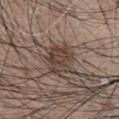This lesion was catalogued during total-body skin photography and was not selected for biopsy. An algorithmic analysis of the crop reported a lesion color around L≈41 a*≈15 b*≈22 in CIELAB, a lesion–skin lightness drop of about 9, and a lesion-to-skin contrast of about 8 (normalized; higher = more distinct). It also reported a border-irregularity rating of about 3/10, internal color variation of about 3.5 on a 0–10 scale, and a peripheral color-asymmetry measure near 1.5. It also reported a nevus-likeness score of about 80/100 and a lesion-detection confidence of about 100/100. A male patient in their mid- to late 70s. Imaged with white-light lighting. A 15 mm close-up extracted from a 3D total-body photography capture. From the abdomen. The lesion's longest dimension is about 4 mm.